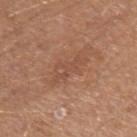Captured during whole-body skin photography for melanoma surveillance; the lesion was not biopsied.
The lesion is located on the left upper arm.
The tile uses white-light illumination.
A close-up tile cropped from a whole-body skin photograph, about 15 mm across.
A male subject, in their 40s.